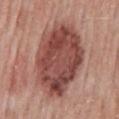Clinical impression: Recorded during total-body skin imaging; not selected for excision or biopsy. Background: The subject is a male approximately 50 years of age. The recorded lesion diameter is about 9.5 mm. On the mid back. Cropped from a whole-body photographic skin survey; the tile spans about 15 mm. Imaged with white-light lighting.